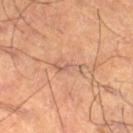follow-up: imaged on a skin check; not biopsied
body site: the right thigh
image: total-body-photography crop, ~15 mm field of view
size: ~3.5 mm (longest diameter)
lighting: cross-polarized
subject: male, aged 53 to 57